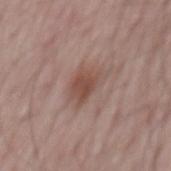Assessment:
Recorded during total-body skin imaging; not selected for excision or biopsy.
Clinical summary:
Cropped from a whole-body photographic skin survey; the tile spans about 15 mm. A male subject, about 65 years old. Longest diameter approximately 4.5 mm. From the mid back. The tile uses white-light illumination.